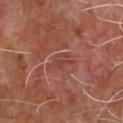The lesion was tiled from a total-body skin photograph and was not biopsied. An algorithmic analysis of the crop reported a mean CIELAB color near L≈41 a*≈26 b*≈27, about 6 CIELAB-L* units darker than the surrounding skin, and a normalized border contrast of about 5.5. And it measured a border-irregularity rating of about 7/10 and a color-variation rating of about 2/10. The analysis additionally found an automated nevus-likeness rating near 0 out of 100 and a lesion-detection confidence of about 80/100. A male subject aged approximately 65. A roughly 15 mm field-of-view crop from a total-body skin photograph. From the chest. The recorded lesion diameter is about 4 mm.